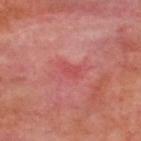image-analysis metrics: an automated nevus-likeness rating near 0 out of 100 and lesion-presence confidence of about 95/100 | lighting: cross-polarized illumination | image: 15 mm crop, total-body photography | patient: male, aged approximately 50 | anatomic site: the back.